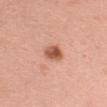Acquisition and patient details: The lesion's longest dimension is about 3 mm. A 15 mm close-up tile from a total-body photography series done for melanoma screening. A female patient about 50 years old. Captured under white-light illumination. On the left upper arm.